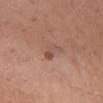biopsy_status: not biopsied; imaged during a skin examination
image:
  source: total-body photography crop
  field_of_view_mm: 15
lighting: white-light
site: chest
patient:
  sex: male
  age_approx: 70
lesion_size:
  long_diameter_mm_approx: 3.5